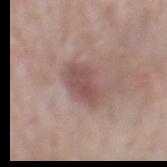– follow-up — imaged on a skin check; not biopsied
– location — the mid back
– size — about 5 mm
– subject — male, aged 53 to 57
– image-analysis metrics — a mean CIELAB color near L≈51 a*≈19 b*≈20 and a lesion–skin lightness drop of about 10; border irregularity of about 3.5 on a 0–10 scale, a color-variation rating of about 2.5/10, and radial color variation of about 1; lesion-presence confidence of about 95/100
– illumination — white-light illumination
– image — total-body-photography crop, ~15 mm field of view